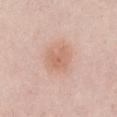| feature | finding |
|---|---|
| biopsy status | total-body-photography surveillance lesion; no biopsy |
| location | the abdomen |
| acquisition | ~15 mm crop, total-body skin-cancer survey |
| lighting | white-light |
| patient | female, approximately 60 years of age |
| image-analysis metrics | a lesion area of about 6.5 mm² and an eccentricity of roughly 0.5; an average lesion color of about L≈64 a*≈22 b*≈30 (CIELAB), roughly 7 lightness units darker than nearby skin, and a lesion-to-skin contrast of about 5.5 (normalized; higher = more distinct); a nevus-likeness score of about 15/100 |